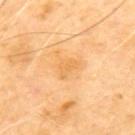Q: Was a biopsy performed?
A: catalogued during a skin exam; not biopsied
Q: Where on the body is the lesion?
A: the upper back
Q: Patient demographics?
A: male, aged around 70
Q: What is the lesion's diameter?
A: about 2.5 mm
Q: Automated lesion metrics?
A: a footprint of about 3.5 mm², a shape eccentricity near 0.8, and a shape-asymmetry score of about 0.45 (0 = symmetric); a lesion color around L≈69 a*≈22 b*≈48 in CIELAB, about 6 CIELAB-L* units darker than the surrounding skin, and a normalized lesion–skin contrast near 5; a border-irregularity rating of about 5/10, a color-variation rating of about 0/10, and radial color variation of about 0; a nevus-likeness score of about 0/100 and a lesion-detection confidence of about 100/100
Q: How was the tile lit?
A: cross-polarized
Q: What kind of image is this?
A: 15 mm crop, total-body photography